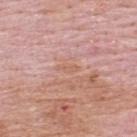Clinical impression:
Part of a total-body skin-imaging series; this lesion was reviewed on a skin check and was not flagged for biopsy.
Context:
Captured under white-light illumination. Measured at roughly 3 mm in maximum diameter. A lesion tile, about 15 mm wide, cut from a 3D total-body photograph. A male subject aged 63–67. On the upper back.